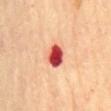subject = female, aged 68–72 | anatomic site = the mid back | acquisition = ~15 mm tile from a whole-body skin photo | illumination = cross-polarized | diameter = ~3 mm (longest diameter) | automated metrics = an average lesion color of about L≈56 a*≈43 b*≈35 (CIELAB), a lesion–skin lightness drop of about 24, and a normalized border contrast of about 14.